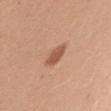<record>
<biopsy_status>not biopsied; imaged during a skin examination</biopsy_status>
<lighting>white-light</lighting>
<patient>
  <sex>female</sex>
  <age_approx>45</age_approx>
</patient>
<image>
  <source>total-body photography crop</source>
  <field_of_view_mm>15</field_of_view_mm>
</image>
<site>left upper arm</site>
<automated_metrics>
  <shape_asymmetry>0.15</shape_asymmetry>
  <nevus_likeness_0_100>95</nevus_likeness_0_100>
  <lesion_detection_confidence_0_100>100</lesion_detection_confidence_0_100>
</automated_metrics>
<lesion_size>
  <long_diameter_mm_approx>3.0</long_diameter_mm_approx>
</lesion_size>
</record>